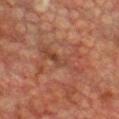  biopsy_status: not biopsied; imaged during a skin examination
  patient:
    sex: male
    age_approx: 75
  image:
    source: total-body photography crop
    field_of_view_mm: 15
  site: front of the torso
  automated_metrics:
    area_mm2_approx: 15.0
    eccentricity: 0.85
    shape_asymmetry: 0.45
    cielab_L: 37
    cielab_a: 21
    cielab_b: 26
    vs_skin_darker_L: 5.0
    vs_skin_contrast_norm: 4.5